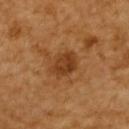biopsy status: imaged on a skin check; not biopsied
lesion size: ≈3.5 mm
acquisition: 15 mm crop, total-body photography
illumination: cross-polarized
patient: female, aged around 55
site: the upper back